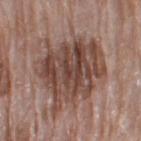The lesion-visualizer software estimated radial color variation of about 2.5. And it measured an automated nevus-likeness rating near 40 out of 100 and a detector confidence of about 100 out of 100 that the crop contains a lesion.
From the right thigh.
About 8.5 mm across.
A 15 mm crop from a total-body photograph taken for skin-cancer surveillance.
A female patient, aged 73 to 77.
This is a white-light tile.